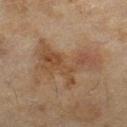Context: Cropped from a whole-body photographic skin survey; the tile spans about 15 mm. A female patient about 60 years old. The lesion is located on the right lower leg.